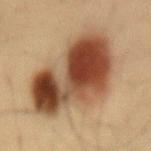| key | value |
|---|---|
| biopsy status | no biopsy performed (imaged during a skin exam) |
| body site | the lower back |
| image | ~15 mm crop, total-body skin-cancer survey |
| subject | male, approximately 35 years of age |
| lesion size | ≈9 mm |